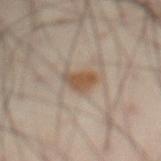{"biopsy_status": "not biopsied; imaged during a skin examination", "lesion_size": {"long_diameter_mm_approx": 3.0}, "site": "front of the torso", "image": {"source": "total-body photography crop", "field_of_view_mm": 15}, "patient": {"sex": "male", "age_approx": 55}}